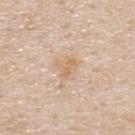Case summary:
– follow-up · imaged on a skin check; not biopsied
– acquisition · 15 mm crop, total-body photography
– site · the back
– patient · male, in their mid-40s